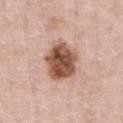– workup — catalogued during a skin exam; not biopsied
– location — the front of the torso
– imaging modality — ~15 mm tile from a whole-body skin photo
– tile lighting — white-light illumination
– subject — male, aged approximately 50
– automated metrics — a lesion area of about 16 mm², an outline eccentricity of about 0.5 (0 = round, 1 = elongated), and two-axis asymmetry of about 0.2; a mean CIELAB color near L≈53 a*≈22 b*≈29, about 18 CIELAB-L* units darker than the surrounding skin, and a lesion-to-skin contrast of about 12 (normalized; higher = more distinct)
– diameter — ~5 mm (longest diameter)